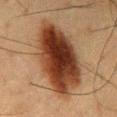Clinical impression:
The lesion was photographed on a routine skin check and not biopsied; there is no pathology result.
Clinical summary:
The recorded lesion diameter is about 10.5 mm. On the back. The subject is a male aged around 60. The tile uses cross-polarized illumination. A close-up tile cropped from a whole-body skin photograph, about 15 mm across.